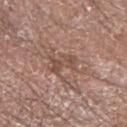The lesion was tiled from a total-body skin photograph and was not biopsied. Automated tile analysis of the lesion measured an eccentricity of roughly 0.8. The analysis additionally found a lesion color around L≈49 a*≈19 b*≈25 in CIELAB, a lesion–skin lightness drop of about 8, and a normalized lesion–skin contrast near 6. Cropped from a whole-body photographic skin survey; the tile spans about 15 mm. This is a white-light tile. Located on the right forearm. A male subject, about 65 years old. The recorded lesion diameter is about 3.5 mm.